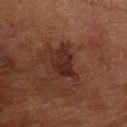<lesion>
  <biopsy_status>not biopsied; imaged during a skin examination</biopsy_status>
  <lighting>cross-polarized</lighting>
  <image>
    <source>total-body photography crop</source>
    <field_of_view_mm>15</field_of_view_mm>
  </image>
  <patient>
    <sex>male</sex>
    <age_approx>65</age_approx>
  </patient>
  <site>left arm</site>
  <lesion_size>
    <long_diameter_mm_approx>4.0</long_diameter_mm_approx>
  </lesion_size>
</lesion>